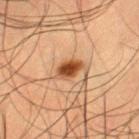This is a cross-polarized tile.
An algorithmic analysis of the crop reported an area of roughly 5 mm², an outline eccentricity of about 0.7 (0 = round, 1 = elongated), and a shape-asymmetry score of about 0.3 (0 = symmetric). And it measured a lesion color around L≈38 a*≈21 b*≈32 in CIELAB, roughly 14 lightness units darker than nearby skin, and a lesion-to-skin contrast of about 11.5 (normalized; higher = more distinct). The analysis additionally found a border-irregularity index near 2.5/10, internal color variation of about 3.5 on a 0–10 scale, and a peripheral color-asymmetry measure near 1. The software also gave an automated nevus-likeness rating near 100 out of 100.
A male subject, aged 48–52.
A lesion tile, about 15 mm wide, cut from a 3D total-body photograph.
On the left thigh.
The lesion's longest dimension is about 3 mm.